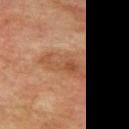The lesion was tiled from a total-body skin photograph and was not biopsied. The tile uses cross-polarized illumination. Measured at roughly 5.5 mm in maximum diameter. The patient is a male approximately 75 years of age. Located on the mid back. A 15 mm close-up tile from a total-body photography series done for melanoma screening.